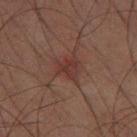A region of skin cropped from a whole-body photographic capture, roughly 15 mm wide.
Located on the leg.
The patient is a male aged 58–62.
Approximately 3 mm at its widest.
Captured under cross-polarized illumination.